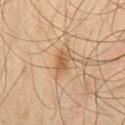Captured during whole-body skin photography for melanoma surveillance; the lesion was not biopsied.
The lesion is located on the right thigh.
This image is a 15 mm lesion crop taken from a total-body photograph.
The lesion-visualizer software estimated a mean CIELAB color near L≈46 a*≈16 b*≈30 and roughly 8 lightness units darker than nearby skin. And it measured a border-irregularity rating of about 2.5/10, a within-lesion color-variation index near 2/10, and radial color variation of about 0.5. The analysis additionally found an automated nevus-likeness rating near 55 out of 100 and a lesion-detection confidence of about 100/100.
About 3 mm across.
A male subject, aged approximately 45.